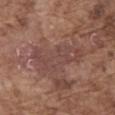Findings:
* biopsy status · total-body-photography surveillance lesion; no biopsy
* anatomic site · the abdomen
* imaging modality · total-body-photography crop, ~15 mm field of view
* patient · male, aged around 75